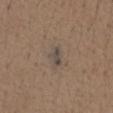The lesion was tiled from a total-body skin photograph and was not biopsied. This is a white-light tile. A male patient aged 73–77. A region of skin cropped from a whole-body photographic capture, roughly 15 mm wide. Located on the abdomen. Automated image analysis of the tile measured a footprint of about 2.5 mm² and a shape eccentricity near 0.85. And it measured a mean CIELAB color near L≈44 a*≈9 b*≈19 and a normalized lesion–skin contrast near 8. The analysis additionally found a border-irregularity rating of about 3/10, internal color variation of about 1 on a 0–10 scale, and a peripheral color-asymmetry measure near 0.5.